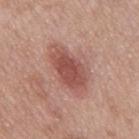The lesion was tiled from a total-body skin photograph and was not biopsied.
About 5.5 mm across.
The lesion is on the upper back.
Automated tile analysis of the lesion measured about 12 CIELAB-L* units darker than the surrounding skin and a lesion-to-skin contrast of about 8 (normalized; higher = more distinct). The analysis additionally found border irregularity of about 2 on a 0–10 scale, a within-lesion color-variation index near 3/10, and radial color variation of about 1. The software also gave a classifier nevus-likeness of about 95/100 and a detector confidence of about 100 out of 100 that the crop contains a lesion.
A male subject aged approximately 55.
A 15 mm close-up tile from a total-body photography series done for melanoma screening.
This is a white-light tile.